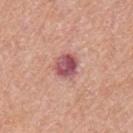Clinical impression:
This lesion was catalogued during total-body skin photography and was not selected for biopsy.
Background:
The patient is a male aged 58–62. The lesion is on the upper back. A 15 mm crop from a total-body photograph taken for skin-cancer surveillance. This is a white-light tile.